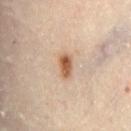notes: imaged on a skin check; not biopsied | diameter: ~3 mm (longest diameter) | illumination: cross-polarized | subject: female, aged 58 to 62 | acquisition: total-body-photography crop, ~15 mm field of view | body site: the lower back.